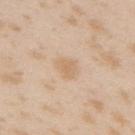This lesion was catalogued during total-body skin photography and was not selected for biopsy.